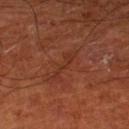Impression: Captured during whole-body skin photography for melanoma surveillance; the lesion was not biopsied. Clinical summary: Located on the left lower leg. A region of skin cropped from a whole-body photographic capture, roughly 15 mm wide. Approximately 4.5 mm at its widest. The subject is a male aged around 65.